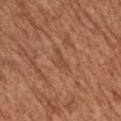Findings:
- follow-up — imaged on a skin check; not biopsied
- patient — female, in their mid- to late 70s
- size — ≈3 mm
- body site — the arm
- image source — ~15 mm tile from a whole-body skin photo